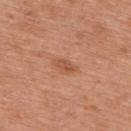This lesion was catalogued during total-body skin photography and was not selected for biopsy. The lesion-visualizer software estimated a shape eccentricity near 0.9. The analysis additionally found a border-irregularity index near 3/10 and a within-lesion color-variation index near 0.5/10. And it measured a detector confidence of about 100 out of 100 that the crop contains a lesion. Approximately 2.5 mm at its widest. Imaged with white-light lighting. The lesion is on the back. Cropped from a total-body skin-imaging series; the visible field is about 15 mm. A male subject approximately 70 years of age.